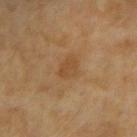Case summary:
* biopsy status: catalogued during a skin exam; not biopsied
* imaging modality: total-body-photography crop, ~15 mm field of view
* body site: the left upper arm
* subject: female, roughly 60 years of age
* lighting: cross-polarized illumination
* size: ≈2.5 mm
* image-analysis metrics: an area of roughly 4 mm², an outline eccentricity of about 0.75 (0 = round, 1 = elongated), and a symmetry-axis asymmetry near 0.25; about 6 CIELAB-L* units darker than the surrounding skin and a lesion-to-skin contrast of about 5.5 (normalized; higher = more distinct); a nevus-likeness score of about 0/100